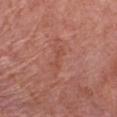Part of a total-body skin-imaging series; this lesion was reviewed on a skin check and was not flagged for biopsy. Automated tile analysis of the lesion measured border irregularity of about 5.5 on a 0–10 scale, a color-variation rating of about 0/10, and peripheral color asymmetry of about 0. A 15 mm crop from a total-body photograph taken for skin-cancer surveillance. Captured under white-light illumination. A male patient aged approximately 80. Located on the chest. The recorded lesion diameter is about 3 mm.